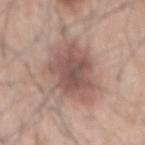Case summary:
– biopsy status — imaged on a skin check; not biopsied
– imaging modality — ~15 mm tile from a whole-body skin photo
– tile lighting — white-light
– patient — male, in their mid- to late 40s
– lesion diameter — ~8 mm (longest diameter)
– automated metrics — a lesion–skin lightness drop of about 12 and a normalized lesion–skin contrast near 8; a border-irregularity index near 3.5/10, internal color variation of about 4 on a 0–10 scale, and a peripheral color-asymmetry measure near 1.5
– anatomic site — the back